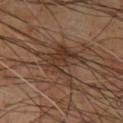Impression: Imaged during a routine full-body skin examination; the lesion was not biopsied and no histopathology is available. Acquisition and patient details: Longest diameter approximately 6.5 mm. From the upper back. The patient is a male aged around 60. The total-body-photography lesion software estimated about 8 CIELAB-L* units darker than the surrounding skin and a normalized border contrast of about 7.5. The analysis additionally found internal color variation of about 4.5 on a 0–10 scale and radial color variation of about 1.5. A 15 mm crop from a total-body photograph taken for skin-cancer surveillance. Imaged with cross-polarized lighting.